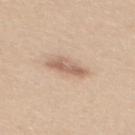follow-up: total-body-photography surveillance lesion; no biopsy | location: the back | lesion diameter: ≈4 mm | tile lighting: white-light illumination | subject: female, in their mid- to late 40s | imaging modality: total-body-photography crop, ~15 mm field of view | TBP lesion metrics: an area of roughly 6.5 mm², a shape eccentricity near 0.85, and a shape-asymmetry score of about 0.25 (0 = symmetric); an average lesion color of about L≈62 a*≈18 b*≈29 (CIELAB) and roughly 11 lightness units darker than nearby skin; a border-irregularity rating of about 3/10 and peripheral color asymmetry of about 1.5.